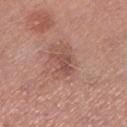  biopsy_status: not biopsied; imaged during a skin examination
  patient:
    sex: male
    age_approx: 50
  image:
    source: total-body photography crop
    field_of_view_mm: 15
  site: left lower leg